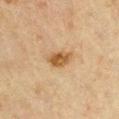Impression:
The lesion was tiled from a total-body skin photograph and was not biopsied.
Acquisition and patient details:
Located on the upper back. Automated tile analysis of the lesion measured a shape eccentricity near 0.75 and a shape-asymmetry score of about 0.25 (0 = symmetric). The software also gave a border-irregularity index near 2.5/10, a within-lesion color-variation index near 3.5/10, and a peripheral color-asymmetry measure near 1.5. A male subject roughly 65 years of age. The lesion's longest dimension is about 3 mm. This is a cross-polarized tile. Cropped from a total-body skin-imaging series; the visible field is about 15 mm.